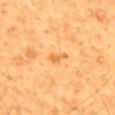Captured during whole-body skin photography for melanoma surveillance; the lesion was not biopsied.
Approximately 3 mm at its widest.
On the back.
An algorithmic analysis of the crop reported an area of roughly 2.5 mm², an outline eccentricity of about 0.9 (0 = round, 1 = elongated), and a symmetry-axis asymmetry near 0.4. It also reported an average lesion color of about L≈65 a*≈24 b*≈48 (CIELAB), about 9 CIELAB-L* units darker than the surrounding skin, and a lesion-to-skin contrast of about 6 (normalized; higher = more distinct). And it measured an automated nevus-likeness rating near 0 out of 100.
A roughly 15 mm field-of-view crop from a total-body skin photograph.
A male patient approximately 60 years of age.